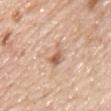Captured during whole-body skin photography for melanoma surveillance; the lesion was not biopsied. A 15 mm close-up tile from a total-body photography series done for melanoma screening. The lesion is on the chest. The subject is a male about 45 years old. Automated image analysis of the tile measured a footprint of about 8.5 mm² and a shape eccentricity near 0.7. Imaged with white-light lighting. Longest diameter approximately 4 mm.